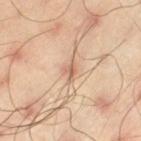Assessment: The lesion was tiled from a total-body skin photograph and was not biopsied. Context: The total-body-photography lesion software estimated an average lesion color of about L≈63 a*≈19 b*≈31 (CIELAB), roughly 10 lightness units darker than nearby skin, and a lesion-to-skin contrast of about 6.5 (normalized; higher = more distinct). The software also gave border irregularity of about 3 on a 0–10 scale, a color-variation rating of about 3.5/10, and a peripheral color-asymmetry measure near 1.5. A close-up tile cropped from a whole-body skin photograph, about 15 mm across. Located on the left thigh. The tile uses cross-polarized illumination. A male patient aged 43–47. The lesion's longest dimension is about 2.5 mm.